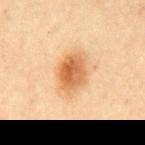This lesion was catalogued during total-body skin photography and was not selected for biopsy.
Cropped from a whole-body photographic skin survey; the tile spans about 15 mm.
From the mid back.
A male patient aged approximately 45.